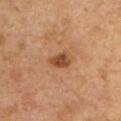biopsy status=imaged on a skin check; not biopsied | image=~15 mm crop, total-body skin-cancer survey | image-analysis metrics=a footprint of about 4 mm² and a shape eccentricity near 0.6; a border-irregularity index near 3.5/10 and a color-variation rating of about 2/10 | patient=male, about 50 years old | tile lighting=cross-polarized illumination | anatomic site=the chest | lesion diameter=~2.5 mm (longest diameter).